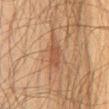{"biopsy_status": "not biopsied; imaged during a skin examination", "image": {"source": "total-body photography crop", "field_of_view_mm": 15}, "lesion_size": {"long_diameter_mm_approx": 3.0}, "patient": {"sex": "male", "age_approx": 60}, "site": "chest"}